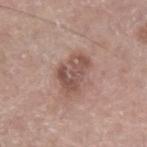Recorded during total-body skin imaging; not selected for excision or biopsy. A 15 mm crop from a total-body photograph taken for skin-cancer surveillance. The lesion is located on the left lower leg. An algorithmic analysis of the crop reported lesion-presence confidence of about 100/100. A male subject in their mid- to late 60s. The tile uses white-light illumination.